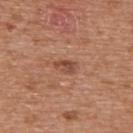Impression:
No biopsy was performed on this lesion — it was imaged during a full skin examination and was not determined to be concerning.
Background:
An algorithmic analysis of the crop reported a footprint of about 3.5 mm², an eccentricity of roughly 0.8, and a symmetry-axis asymmetry near 0.3. The analysis additionally found a lesion–skin lightness drop of about 10 and a lesion-to-skin contrast of about 7 (normalized; higher = more distinct). The analysis additionally found border irregularity of about 3.5 on a 0–10 scale, a color-variation rating of about 2/10, and a peripheral color-asymmetry measure near 0.5. It also reported an automated nevus-likeness rating near 5 out of 100. The patient is a male in their mid-60s. The lesion is located on the upper back. About 2.5 mm across. A close-up tile cropped from a whole-body skin photograph, about 15 mm across.